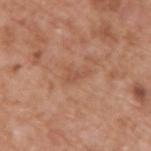Case summary:
– follow-up · imaged on a skin check; not biopsied
– tile lighting · white-light
– subject · male, aged 63–67
– size · about 2.5 mm
– location · the back
– image · 15 mm crop, total-body photography